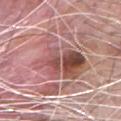Context:
A male subject, about 80 years old. The lesion is located on the chest. This image is a 15 mm lesion crop taken from a total-body photograph.